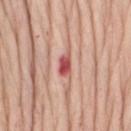Clinical impression:
Recorded during total-body skin imaging; not selected for excision or biopsy.
Image and clinical context:
A roughly 15 mm field-of-view crop from a total-body skin photograph. Captured under white-light illumination. A female subject approximately 75 years of age. Located on the lower back.